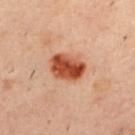Q: Was a biopsy performed?
A: imaged on a skin check; not biopsied
Q: What is the imaging modality?
A: ~15 mm tile from a whole-body skin photo
Q: How large is the lesion?
A: ~4.5 mm (longest diameter)
Q: Lesion location?
A: the back
Q: Illumination type?
A: cross-polarized illumination
Q: What are the patient's age and sex?
A: male, roughly 40 years of age